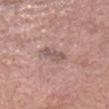Image and clinical context: Automated image analysis of the tile measured a lesion area of about 5 mm² and a shape-asymmetry score of about 0.3 (0 = symmetric). It also reported a lesion color around L≈55 a*≈18 b*≈21 in CIELAB and a normalized border contrast of about 6. A female subject aged 63 to 67. Captured under white-light illumination. A 15 mm close-up extracted from a 3D total-body photography capture. Longest diameter approximately 3.5 mm. The lesion is located on the head or neck.